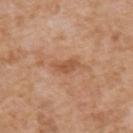Notes:
• follow-up — imaged on a skin check; not biopsied
• illumination — white-light
• TBP lesion metrics — a footprint of about 4.5 mm², a shape eccentricity near 0.9, and a symmetry-axis asymmetry near 0.35; border irregularity of about 3.5 on a 0–10 scale and radial color variation of about 0.5
• diameter — ≈4 mm
• imaging modality — total-body-photography crop, ~15 mm field of view
• site — the upper back
• patient — male, in their 60s